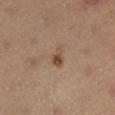biopsy_status: not biopsied; imaged during a skin examination
image:
  source: total-body photography crop
  field_of_view_mm: 15
lighting: cross-polarized
site: right lower leg
lesion_size:
  long_diameter_mm_approx: 2.5
patient:
  sex: female
  age_approx: 35